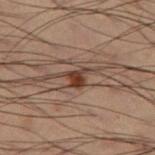This lesion was catalogued during total-body skin photography and was not selected for biopsy.
This is a cross-polarized tile.
Measured at roughly 2.5 mm in maximum diameter.
A male subject aged approximately 55.
Cropped from a total-body skin-imaging series; the visible field is about 15 mm.
Located on the leg.